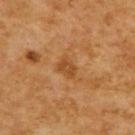notes=imaged on a skin check; not biopsied | lesion diameter=≈2.5 mm | tile lighting=cross-polarized | location=the upper back | image-analysis metrics=an average lesion color of about L≈44 a*≈22 b*≈39 (CIELAB) and a normalized lesion–skin contrast near 6; border irregularity of about 3 on a 0–10 scale, a color-variation rating of about 1.5/10, and radial color variation of about 0.5 | subject=male, aged approximately 60 | image source=~15 mm tile from a whole-body skin photo.